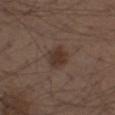The lesion was photographed on a routine skin check and not biopsied; there is no pathology result. Imaged with white-light lighting. The subject is a male aged approximately 40. Automated tile analysis of the lesion measured a footprint of about 6 mm², an eccentricity of roughly 0.5, and two-axis asymmetry of about 0.2. The analysis additionally found an average lesion color of about L≈33 a*≈15 b*≈22 (CIELAB) and about 8 CIELAB-L* units darker than the surrounding skin. It also reported a border-irregularity rating of about 2/10 and peripheral color asymmetry of about 1. On the arm. Longest diameter approximately 3 mm. A 15 mm crop from a total-body photograph taken for skin-cancer surveillance.